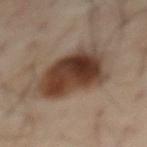* biopsy status — no biopsy performed (imaged during a skin exam)
* anatomic site — the front of the torso
* patient — male, in their mid- to late 60s
* lesion diameter — about 6.5 mm
* TBP lesion metrics — about 15 CIELAB-L* units darker than the surrounding skin and a normalized border contrast of about 12.5; a border-irregularity rating of about 3/10, a color-variation rating of about 8/10, and radial color variation of about 3
* acquisition — ~15 mm tile from a whole-body skin photo
* illumination — cross-polarized